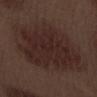workup=imaged on a skin check; not biopsied
lighting=white-light illumination
patient=male, aged 68 to 72
image=~15 mm crop, total-body skin-cancer survey
lesion size=about 9.5 mm
anatomic site=the arm